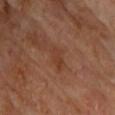The lesion was photographed on a routine skin check and not biopsied; there is no pathology result. The lesion is located on the front of the torso. A lesion tile, about 15 mm wide, cut from a 3D total-body photograph. A male patient about 60 years old.